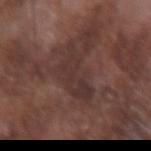Q: Was this lesion biopsied?
A: no biopsy performed (imaged during a skin exam)
Q: What lighting was used for the tile?
A: white-light
Q: Lesion location?
A: the right forearm
Q: How was this image acquired?
A: ~15 mm crop, total-body skin-cancer survey
Q: Who is the patient?
A: male, roughly 75 years of age
Q: How large is the lesion?
A: ≈5.5 mm